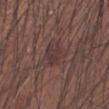biopsy_status: not biopsied; imaged during a skin examination
site: left forearm
image:
  source: total-body photography crop
  field_of_view_mm: 15
patient:
  sex: male
  age_approx: 35